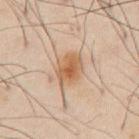Impression:
Recorded during total-body skin imaging; not selected for excision or biopsy.
Background:
A male subject, in their mid-50s. The tile uses cross-polarized illumination. On the abdomen. Cropped from a total-body skin-imaging series; the visible field is about 15 mm. Measured at roughly 4 mm in maximum diameter.